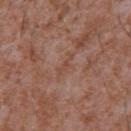workup: imaged on a skin check; not biopsied
image source: ~15 mm crop, total-body skin-cancer survey
lesion diameter: ~3 mm (longest diameter)
TBP lesion metrics: a footprint of about 2.5 mm² and two-axis asymmetry of about 0.45; roughly 5 lightness units darker than nearby skin and a normalized border contrast of about 5.5; a nevus-likeness score of about 0/100
lighting: white-light illumination
subject: male, in their mid-40s
location: the chest